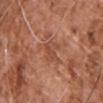Q: Is there a histopathology result?
A: total-body-photography surveillance lesion; no biopsy
Q: What lighting was used for the tile?
A: white-light illumination
Q: Automated lesion metrics?
A: a mean CIELAB color near L≈49 a*≈25 b*≈32, roughly 8 lightness units darker than nearby skin, and a lesion-to-skin contrast of about 6 (normalized; higher = more distinct)
Q: How large is the lesion?
A: about 4 mm
Q: What is the imaging modality?
A: 15 mm crop, total-body photography
Q: Where on the body is the lesion?
A: the chest
Q: Who is the patient?
A: male, about 75 years old